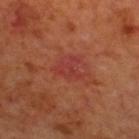Q: What is the lesion's diameter?
A: ≈3 mm
Q: What are the patient's age and sex?
A: male, in their 70s
Q: How was this image acquired?
A: ~15 mm crop, total-body skin-cancer survey
Q: Where on the body is the lesion?
A: the upper back